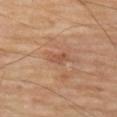notes=imaged on a skin check; not biopsied
acquisition=~15 mm tile from a whole-body skin photo
automated lesion analysis=an area of roughly 3.5 mm² and an eccentricity of roughly 0.85; an average lesion color of about L≈51 a*≈22 b*≈31 (CIELAB), roughly 7 lightness units darker than nearby skin, and a normalized lesion–skin contrast near 5.5; an automated nevus-likeness rating near 0 out of 100 and lesion-presence confidence of about 100/100
lesion diameter=~3 mm (longest diameter)
subject=male, approximately 70 years of age
body site=the left thigh
illumination=cross-polarized